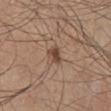follow-up: total-body-photography surveillance lesion; no biopsy
TBP lesion metrics: an area of roughly 3.5 mm², an outline eccentricity of about 0.65 (0 = round, 1 = elongated), and two-axis asymmetry of about 0.35; border irregularity of about 3 on a 0–10 scale, internal color variation of about 3 on a 0–10 scale, and peripheral color asymmetry of about 1
location: the leg
subject: male, aged 43 to 47
tile lighting: white-light illumination
lesion size: about 2.5 mm
image: 15 mm crop, total-body photography